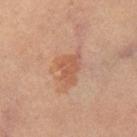The lesion was photographed on a routine skin check and not biopsied; there is no pathology result.
A 15 mm close-up tile from a total-body photography series done for melanoma screening.
The tile uses cross-polarized illumination.
The lesion's longest dimension is about 3.5 mm.
The subject is a female aged approximately 65.
Located on the leg.